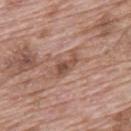Background: Cropped from a total-body skin-imaging series; the visible field is about 15 mm. A male patient, roughly 70 years of age. The recorded lesion diameter is about 3.5 mm. The tile uses white-light illumination. An algorithmic analysis of the crop reported a lesion area of about 5 mm², a shape eccentricity near 0.9, and two-axis asymmetry of about 0.4. The analysis additionally found an average lesion color of about L≈50 a*≈21 b*≈27 (CIELAB) and about 10 CIELAB-L* units darker than the surrounding skin. It also reported a lesion-detection confidence of about 100/100. Located on the back.